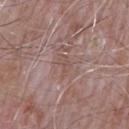Recorded during total-body skin imaging; not selected for excision or biopsy. A 15 mm close-up tile from a total-body photography series done for melanoma screening. A male patient aged 63 to 67. The tile uses white-light illumination. The lesion-visualizer software estimated a footprint of about 3.5 mm² and two-axis asymmetry of about 0.45. And it measured a color-variation rating of about 2/10 and a peripheral color-asymmetry measure near 0.5. And it measured a classifier nevus-likeness of about 0/100. The lesion is located on the arm. The lesion's longest dimension is about 3 mm.